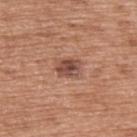| field | value |
|---|---|
| follow-up | no biopsy performed (imaged during a skin exam) |
| subject | male, in their mid-70s |
| diameter | about 3 mm |
| imaging modality | total-body-photography crop, ~15 mm field of view |
| site | the upper back |